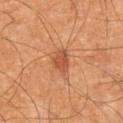notes — imaged on a skin check; not biopsied
automated lesion analysis — a lesion area of about 4.5 mm² and a shape eccentricity near 0.55; a within-lesion color-variation index near 2/10 and a peripheral color-asymmetry measure near 1; a nevus-likeness score of about 90/100 and a detector confidence of about 100 out of 100 that the crop contains a lesion
lesion diameter — ~2.5 mm (longest diameter)
subject — male, aged approximately 65
location — the left lower leg
acquisition — ~15 mm tile from a whole-body skin photo
illumination — cross-polarized